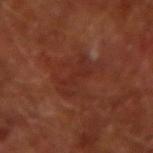The lesion was photographed on a routine skin check and not biopsied; there is no pathology result. Located on the right forearm. This image is a 15 mm lesion crop taken from a total-body photograph. The subject is a male aged 63–67. This is a cross-polarized tile. Measured at roughly 4.5 mm in maximum diameter.